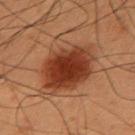follow-up: imaged on a skin check; not biopsied | image-analysis metrics: an area of roughly 25 mm², an outline eccentricity of about 0.65 (0 = round, 1 = elongated), and a symmetry-axis asymmetry near 0.15; border irregularity of about 2 on a 0–10 scale, a within-lesion color-variation index near 4.5/10, and radial color variation of about 1; a classifier nevus-likeness of about 100/100 and a detector confidence of about 100 out of 100 that the crop contains a lesion | subject: male, aged 53–57 | acquisition: 15 mm crop, total-body photography | location: the right upper arm | illumination: cross-polarized illumination.